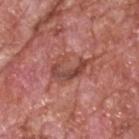The tile uses white-light illumination. A male subject aged around 60. Cropped from a total-body skin-imaging series; the visible field is about 15 mm. Automated tile analysis of the lesion measured roughly 9 lightness units darker than nearby skin and a normalized border contrast of about 7. It also reported a border-irregularity rating of about 7.5/10, a within-lesion color-variation index near 5.5/10, and radial color variation of about 2. About 5 mm across. The lesion is on the head or neck.